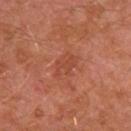Assessment: Part of a total-body skin-imaging series; this lesion was reviewed on a skin check and was not flagged for biopsy. Image and clinical context: The total-body-photography lesion software estimated a lesion color around L≈45 a*≈29 b*≈33 in CIELAB, about 6 CIELAB-L* units darker than the surrounding skin, and a lesion-to-skin contrast of about 4.5 (normalized; higher = more distinct). Imaged with cross-polarized lighting. On the left arm. The recorded lesion diameter is about 3.5 mm. A male patient aged around 30. A 15 mm crop from a total-body photograph taken for skin-cancer surveillance.